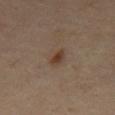No biopsy was performed on this lesion — it was imaged during a full skin examination and was not determined to be concerning.
Located on the abdomen.
Cropped from a whole-body photographic skin survey; the tile spans about 15 mm.
An algorithmic analysis of the crop reported a border-irregularity index near 2/10, internal color variation of about 1 on a 0–10 scale, and radial color variation of about 0. And it measured a classifier nevus-likeness of about 95/100.
The subject is a male about 55 years old.
The recorded lesion diameter is about 2.5 mm.